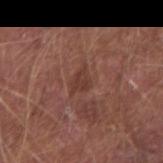Findings:
* follow-up · no biopsy performed (imaged during a skin exam)
* patient · male, in their mid-70s
* imaging modality · total-body-photography crop, ~15 mm field of view
* lesion size · about 3 mm
* site · the right forearm